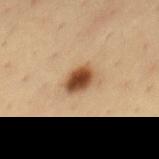The lesion was photographed on a routine skin check and not biopsied; there is no pathology result. About 3 mm across. A 15 mm close-up extracted from a 3D total-body photography capture. A male patient in their mid-30s. The lesion is on the mid back.